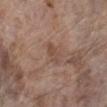Assessment: This lesion was catalogued during total-body skin photography and was not selected for biopsy. Clinical summary: A 15 mm close-up extracted from a 3D total-body photography capture. Automated tile analysis of the lesion measured peripheral color asymmetry of about 0.5. And it measured a nevus-likeness score of about 0/100 and a detector confidence of about 100 out of 100 that the crop contains a lesion. This is a white-light tile. Measured at roughly 3 mm in maximum diameter. From the left lower leg. A female subject about 85 years old or older.